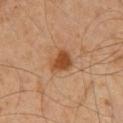notes=catalogued during a skin exam; not biopsied
acquisition=15 mm crop, total-body photography
automated metrics=a footprint of about 7.5 mm² and an eccentricity of roughly 0.65; a border-irregularity index near 2.5/10 and a color-variation rating of about 3.5/10
subject=male, about 60 years old
lesion size=about 3.5 mm
body site=the chest
illumination=cross-polarized